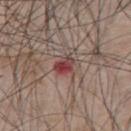{"biopsy_status": "not biopsied; imaged during a skin examination", "image": {"source": "total-body photography crop", "field_of_view_mm": 15}, "patient": {"sex": "male", "age_approx": 45}, "lesion_size": {"long_diameter_mm_approx": 2.5}, "site": "chest"}